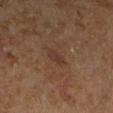Captured during whole-body skin photography for melanoma surveillance; the lesion was not biopsied. The recorded lesion diameter is about 2.5 mm. The total-body-photography lesion software estimated an area of roughly 3.5 mm², an outline eccentricity of about 0.75 (0 = round, 1 = elongated), and a shape-asymmetry score of about 0.3 (0 = symmetric). The analysis additionally found an average lesion color of about L≈32 a*≈16 b*≈24 (CIELAB), about 5 CIELAB-L* units darker than the surrounding skin, and a normalized border contrast of about 5. A male subject, aged approximately 60. The tile uses cross-polarized illumination. The lesion is located on the left lower leg. A 15 mm close-up tile from a total-body photography series done for melanoma screening.